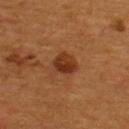workup — catalogued during a skin exam; not biopsied
lighting — cross-polarized
acquisition — 15 mm crop, total-body photography
location — the upper back
subject — male, roughly 55 years of age